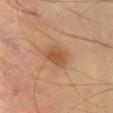Q: Is there a histopathology result?
A: catalogued during a skin exam; not biopsied
Q: How large is the lesion?
A: about 3.5 mm
Q: Illumination type?
A: cross-polarized illumination
Q: What are the patient's age and sex?
A: male, aged 53–57
Q: How was this image acquired?
A: 15 mm crop, total-body photography
Q: Lesion location?
A: the abdomen